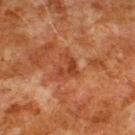{"biopsy_status": "not biopsied; imaged during a skin examination", "image": {"source": "total-body photography crop", "field_of_view_mm": 15}, "patient": {"sex": "male", "age_approx": 80}, "lighting": "cross-polarized", "lesion_size": {"long_diameter_mm_approx": 3.0}, "site": "upper back"}